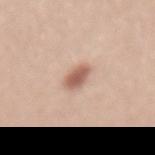follow-up: catalogued during a skin exam; not biopsied | lesion diameter: ≈3 mm | tile lighting: white-light | imaging modality: ~15 mm tile from a whole-body skin photo | automated lesion analysis: a footprint of about 5 mm², an outline eccentricity of about 0.8 (0 = round, 1 = elongated), and two-axis asymmetry of about 0.2; a nevus-likeness score of about 95/100 | location: the mid back | patient: female, aged 33–37.